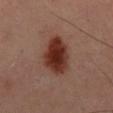A male patient, aged 48 to 52.
The total-body-photography lesion software estimated an average lesion color of about L≈32 a*≈23 b*≈26 (CIELAB) and about 14 CIELAB-L* units darker than the surrounding skin. It also reported internal color variation of about 5 on a 0–10 scale and a peripheral color-asymmetry measure near 1.5.
From the mid back.
Captured under cross-polarized illumination.
The recorded lesion diameter is about 6 mm.
Cropped from a whole-body photographic skin survey; the tile spans about 15 mm.